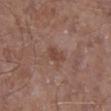follow-up=imaged on a skin check; not biopsied | image source=total-body-photography crop, ~15 mm field of view | anatomic site=the leg | illumination=white-light | subject=male, roughly 45 years of age | size=about 3 mm.